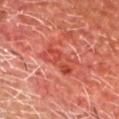Assessment:
No biopsy was performed on this lesion — it was imaged during a full skin examination and was not determined to be concerning.
Background:
The recorded lesion diameter is about 4.5 mm. A male patient approximately 65 years of age. The lesion is located on the head or neck. A region of skin cropped from a whole-body photographic capture, roughly 15 mm wide. The lesion-visualizer software estimated border irregularity of about 3.5 on a 0–10 scale, internal color variation of about 5.5 on a 0–10 scale, and peripheral color asymmetry of about 2. Captured under cross-polarized illumination.